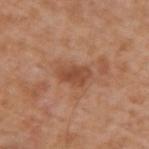Findings:
• workup: total-body-photography surveillance lesion; no biopsy
• subject: male, in their mid- to late 60s
• acquisition: ~15 mm tile from a whole-body skin photo
• site: the upper back